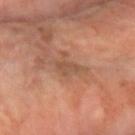Cropped from a total-body skin-imaging series; the visible field is about 15 mm. The total-body-photography lesion software estimated a lesion color around L≈49 a*≈20 b*≈31 in CIELAB and a lesion-to-skin contrast of about 5 (normalized; higher = more distinct). Approximately 3 mm at its widest. The subject is a female aged 63 to 67. The lesion is on the arm.